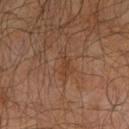Impression:
The lesion was photographed on a routine skin check and not biopsied; there is no pathology result.
Clinical summary:
A male subject, in their mid- to late 60s. Captured under cross-polarized illumination. Measured at roughly 3 mm in maximum diameter. This image is a 15 mm lesion crop taken from a total-body photograph. Automated image analysis of the tile measured roughly 5 lightness units darker than nearby skin and a lesion-to-skin contrast of about 5 (normalized; higher = more distinct). The software also gave a border-irregularity index near 6/10 and peripheral color asymmetry of about 0. And it measured a nevus-likeness score of about 0/100 and lesion-presence confidence of about 95/100. Located on the right upper arm.